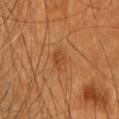This lesion was catalogued during total-body skin photography and was not selected for biopsy. An algorithmic analysis of the crop reported a footprint of about 3.5 mm², a shape eccentricity near 0.75, and a shape-asymmetry score of about 0.25 (0 = symmetric). The software also gave a border-irregularity index near 2.5/10 and internal color variation of about 1.5 on a 0–10 scale. Measured at roughly 2.5 mm in maximum diameter. On the head or neck. A 15 mm close-up extracted from a 3D total-body photography capture. This is a cross-polarized tile. A female subject in their mid- to late 50s.